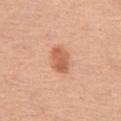Clinical impression: The lesion was tiled from a total-body skin photograph and was not biopsied. Background: On the right thigh. A 15 mm close-up tile from a total-body photography series done for melanoma screening. The subject is a female roughly 65 years of age.